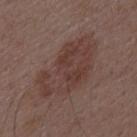Clinical impression:
Captured during whole-body skin photography for melanoma surveillance; the lesion was not biopsied.
Clinical summary:
A close-up tile cropped from a whole-body skin photograph, about 15 mm across. Measured at roughly 9 mm in maximum diameter. The lesion is on the mid back. A male patient roughly 50 years of age. The lesion-visualizer software estimated a color-variation rating of about 4/10 and a peripheral color-asymmetry measure near 1.5. The software also gave a classifier nevus-likeness of about 10/100 and a lesion-detection confidence of about 100/100. This is a white-light tile.